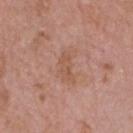Clinical impression: The lesion was photographed on a routine skin check and not biopsied; there is no pathology result. Context: A 15 mm close-up extracted from a 3D total-body photography capture. A male patient, aged 73 to 77. Located on the head or neck. Measured at roughly 3.5 mm in maximum diameter. Captured under white-light illumination.